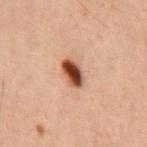{"biopsy_status": "not biopsied; imaged during a skin examination", "lighting": "cross-polarized", "lesion_size": {"long_diameter_mm_approx": 3.5}, "image": {"source": "total-body photography crop", "field_of_view_mm": 15}, "patient": {"sex": "male", "age_approx": 60}, "site": "chest", "automated_metrics": {"area_mm2_approx": 6.0, "eccentricity": 0.75, "vs_skin_darker_L": 17.0, "vs_skin_contrast_norm": 13.5}}